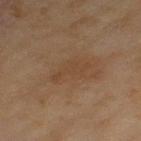{"biopsy_status": "not biopsied; imaged during a skin examination", "patient": {"sex": "female", "age_approx": 60}, "site": "left thigh", "image": {"source": "total-body photography crop", "field_of_view_mm": 15}}